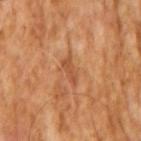Captured under cross-polarized illumination. A male patient, aged around 65. A roughly 15 mm field-of-view crop from a total-body skin photograph. About 3.5 mm across.